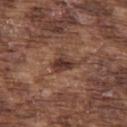Imaged during a routine full-body skin examination; the lesion was not biopsied and no histopathology is available.
An algorithmic analysis of the crop reported an area of roughly 5 mm² and an outline eccentricity of about 0.7 (0 = round, 1 = elongated). It also reported a border-irregularity index near 3.5/10, internal color variation of about 3 on a 0–10 scale, and a peripheral color-asymmetry measure near 1.
Longest diameter approximately 3 mm.
The subject is a male in their mid- to late 70s.
Cropped from a total-body skin-imaging series; the visible field is about 15 mm.
Located on the upper back.
The tile uses white-light illumination.